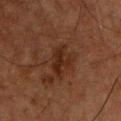No biopsy was performed on this lesion — it was imaged during a full skin examination and was not determined to be concerning.
From the chest.
An algorithmic analysis of the crop reported roughly 6 lightness units darker than nearby skin and a normalized border contrast of about 8. And it measured an automated nevus-likeness rating near 0 out of 100 and lesion-presence confidence of about 100/100.
Cropped from a whole-body photographic skin survey; the tile spans about 15 mm.
Imaged with cross-polarized lighting.
Longest diameter approximately 4.5 mm.
A male subject in their 50s.